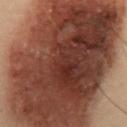patient:
  sex: male
  age_approx: 55
image:
  source: total-body photography crop
  field_of_view_mm: 15
site: abdomen